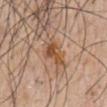Recorded during total-body skin imaging; not selected for excision or biopsy. Cropped from a whole-body photographic skin survey; the tile spans about 15 mm. Captured under white-light illumination. A male subject aged 53 to 57. The lesion is located on the chest.